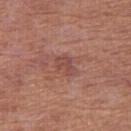Part of a total-body skin-imaging series; this lesion was reviewed on a skin check and was not flagged for biopsy. A roughly 15 mm field-of-view crop from a total-body skin photograph. Automated image analysis of the tile measured a footprint of about 3.5 mm², a shape eccentricity near 0.8, and a symmetry-axis asymmetry near 0.45. And it measured an average lesion color of about L≈47 a*≈24 b*≈25 (CIELAB), a lesion–skin lightness drop of about 7, and a lesion-to-skin contrast of about 5.5 (normalized; higher = more distinct). It also reported a border-irregularity rating of about 4.5/10, internal color variation of about 1.5 on a 0–10 scale, and a peripheral color-asymmetry measure near 0.5. A female patient, in their mid-50s. On the right thigh. Captured under white-light illumination.